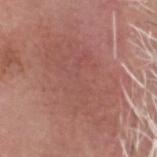Impression: Imaged during a routine full-body skin examination; the lesion was not biopsied and no histopathology is available. Image and clinical context: The lesion's longest dimension is about 13 mm. Cropped from a whole-body photographic skin survey; the tile spans about 15 mm. The subject is a male aged 73–77. From the head or neck. Captured under white-light illumination. Automated tile analysis of the lesion measured a footprint of about 60 mm², an outline eccentricity of about 0.8 (0 = round, 1 = elongated), and a symmetry-axis asymmetry near 0.2. The analysis additionally found a nevus-likeness score of about 0/100 and lesion-presence confidence of about 95/100.